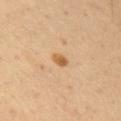Assessment: No biopsy was performed on this lesion — it was imaged during a full skin examination and was not determined to be concerning. Context: A male patient aged 63–67. The lesion is located on the right upper arm. This is a cross-polarized tile. A 15 mm crop from a total-body photograph taken for skin-cancer surveillance.